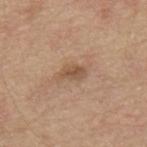The lesion was photographed on a routine skin check and not biopsied; there is no pathology result. Longest diameter approximately 3 mm. A 15 mm close-up extracted from a 3D total-body photography capture. A male patient, aged approximately 70. Located on the mid back. This is a white-light tile.